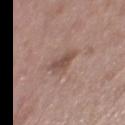Clinical impression:
No biopsy was performed on this lesion — it was imaged during a full skin examination and was not determined to be concerning.
Acquisition and patient details:
The lesion is on the leg. The subject is a male roughly 50 years of age. This is a white-light tile. The lesion-visualizer software estimated an area of roughly 4.5 mm², an eccentricity of roughly 0.65, and a symmetry-axis asymmetry near 0.35. The software also gave an average lesion color of about L≈49 a*≈17 b*≈24 (CIELAB) and a lesion-to-skin contrast of about 6.5 (normalized; higher = more distinct). It also reported a border-irregularity rating of about 3/10, a within-lesion color-variation index near 2/10, and radial color variation of about 0.5. The recorded lesion diameter is about 3 mm. A lesion tile, about 15 mm wide, cut from a 3D total-body photograph.